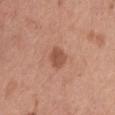The lesion was photographed on a routine skin check and not biopsied; there is no pathology result. The subject is a female aged 43–47. A close-up tile cropped from a whole-body skin photograph, about 15 mm across. Located on the chest.